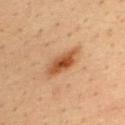Longest diameter approximately 5 mm. A 15 mm crop from a total-body photograph taken for skin-cancer surveillance. The lesion is on the upper back. A male patient, in their mid-30s. Automated image analysis of the tile measured border irregularity of about 3.5 on a 0–10 scale and a peripheral color-asymmetry measure near 1.5. And it measured an automated nevus-likeness rating near 95 out of 100 and a lesion-detection confidence of about 100/100.